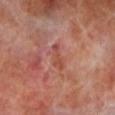workup: no biopsy performed (imaged during a skin exam)
lesion size: ≈3.5 mm
automated lesion analysis: a border-irregularity rating of about 4.5/10, a within-lesion color-variation index near 0/10, and peripheral color asymmetry of about 0; a lesion-detection confidence of about 100/100
patient: male, aged approximately 70
body site: the left lower leg
tile lighting: cross-polarized
imaging modality: ~15 mm crop, total-body skin-cancer survey